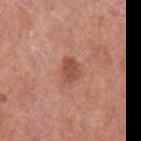Q: Was a biopsy performed?
A: total-body-photography surveillance lesion; no biopsy
Q: How large is the lesion?
A: about 2.5 mm
Q: What kind of image is this?
A: 15 mm crop, total-body photography
Q: What is the anatomic site?
A: the left upper arm
Q: Automated lesion metrics?
A: a shape eccentricity near 0.7; a lesion color around L≈50 a*≈26 b*≈29 in CIELAB and a lesion-to-skin contrast of about 7 (normalized; higher = more distinct); a nevus-likeness score of about 50/100
Q: Patient demographics?
A: male, aged around 70